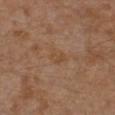Assessment: No biopsy was performed on this lesion — it was imaged during a full skin examination and was not determined to be concerning. Image and clinical context: The lesion's longest dimension is about 2.5 mm. The lesion is located on the left leg. A male subject aged around 30. Cropped from a total-body skin-imaging series; the visible field is about 15 mm.